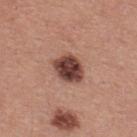No biopsy was performed on this lesion — it was imaged during a full skin examination and was not determined to be concerning. A male patient, about 40 years old. Automated tile analysis of the lesion measured a footprint of about 9.5 mm². The analysis additionally found a border-irregularity index near 1.5/10 and a color-variation rating of about 5/10. The software also gave lesion-presence confidence of about 100/100. A roughly 15 mm field-of-view crop from a total-body skin photograph. Located on the back.